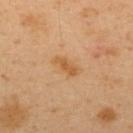Part of a total-body skin-imaging series; this lesion was reviewed on a skin check and was not flagged for biopsy.
Automated tile analysis of the lesion measured an area of roughly 4.5 mm² and an outline eccentricity of about 0.9 (0 = round, 1 = elongated). The analysis additionally found an average lesion color of about L≈58 a*≈21 b*≈42 (CIELAB) and a lesion-to-skin contrast of about 7 (normalized; higher = more distinct).
A 15 mm close-up tile from a total-body photography series done for melanoma screening.
A male patient, aged 38–42.
Located on the upper back.
This is a cross-polarized tile.
Longest diameter approximately 3.5 mm.